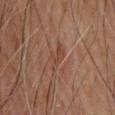{
  "biopsy_status": "not biopsied; imaged during a skin examination",
  "site": "back",
  "image": {
    "source": "total-body photography crop",
    "field_of_view_mm": 15
  },
  "patient": {
    "sex": "male",
    "age_approx": 65
  },
  "automated_metrics": {
    "area_mm2_approx": 4.0,
    "eccentricity": 0.9,
    "nevus_likeness_0_100": 0
  }
}